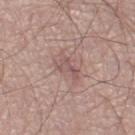Q: Was this lesion biopsied?
A: imaged on a skin check; not biopsied
Q: What kind of image is this?
A: ~15 mm crop, total-body skin-cancer survey
Q: Lesion location?
A: the left leg
Q: How large is the lesion?
A: ~3.5 mm (longest diameter)
Q: Illumination type?
A: white-light illumination
Q: Who is the patient?
A: male, aged approximately 80
Q: Automated lesion metrics?
A: an eccentricity of roughly 0.85 and a symmetry-axis asymmetry near 0.5; about 8 CIELAB-L* units darker than the surrounding skin and a normalized border contrast of about 5.5